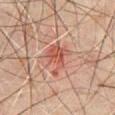Clinical impression: Part of a total-body skin-imaging series; this lesion was reviewed on a skin check and was not flagged for biopsy. Clinical summary: The lesion is on the mid back. A male patient in their 70s. A lesion tile, about 15 mm wide, cut from a 3D total-body photograph. Imaged with cross-polarized lighting.